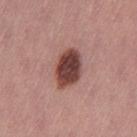Part of a total-body skin-imaging series; this lesion was reviewed on a skin check and was not flagged for biopsy.
A 15 mm close-up tile from a total-body photography series done for melanoma screening.
The subject is a female aged around 55.
The lesion is located on the leg.
This is a white-light tile.
The recorded lesion diameter is about 4.5 mm.